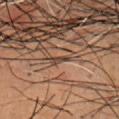Part of a total-body skin-imaging series; this lesion was reviewed on a skin check and was not flagged for biopsy.
From the head or neck.
An algorithmic analysis of the crop reported an eccentricity of roughly 0.95. And it measured border irregularity of about 3.5 on a 0–10 scale, a color-variation rating of about 0/10, and peripheral color asymmetry of about 0. It also reported a nevus-likeness score of about 0/100 and lesion-presence confidence of about 0/100.
A 15 mm crop from a total-body photograph taken for skin-cancer surveillance.
A male patient aged around 55.